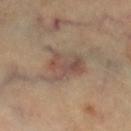Notes:
– tile lighting: cross-polarized illumination
– patient: aged 58 to 62
– lesion diameter: about 5 mm
– body site: the right lower leg
– image source: total-body-photography crop, ~15 mm field of view